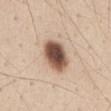Q: Is there a histopathology result?
A: no biopsy performed (imaged during a skin exam)
Q: How large is the lesion?
A: about 4.5 mm
Q: Automated lesion metrics?
A: an area of roughly 12 mm², an eccentricity of roughly 0.75, and a symmetry-axis asymmetry near 0.1; an average lesion color of about L≈52 a*≈18 b*≈27 (CIELAB), about 21 CIELAB-L* units darker than the surrounding skin, and a normalized lesion–skin contrast near 13.5; a border-irregularity rating of about 1/10, a within-lesion color-variation index near 7.5/10, and radial color variation of about 2; a nevus-likeness score of about 90/100 and lesion-presence confidence of about 100/100
Q: Lesion location?
A: the abdomen
Q: How was this image acquired?
A: ~15 mm tile from a whole-body skin photo
Q: How was the tile lit?
A: white-light
Q: Who is the patient?
A: male, roughly 45 years of age